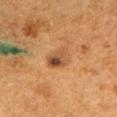biopsy_status: not biopsied; imaged during a skin examination
image:
  source: total-body photography crop
  field_of_view_mm: 15
patient:
  sex: male
  age_approx: 50
lesion_size:
  long_diameter_mm_approx: 3.5
site: left upper arm
lighting: cross-polarized